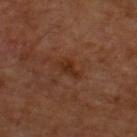{
  "biopsy_status": "not biopsied; imaged during a skin examination",
  "lighting": "cross-polarized",
  "image": {
    "source": "total-body photography crop",
    "field_of_view_mm": 15
  },
  "lesion_size": {
    "long_diameter_mm_approx": 2.5
  },
  "patient": {
    "sex": "male",
    "age_approx": 60
  },
  "automated_metrics": {
    "area_mm2_approx": 3.0,
    "eccentricity": 0.85,
    "cielab_L": 26,
    "cielab_a": 20,
    "cielab_b": 27,
    "vs_skin_darker_L": 6.0,
    "vs_skin_contrast_norm": 7.5
  },
  "site": "upper back"
}